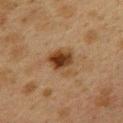- biopsy status: no biopsy performed (imaged during a skin exam)
- subject: female, in their 40s
- site: the back
- imaging modality: total-body-photography crop, ~15 mm field of view